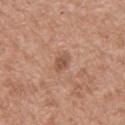biopsy_status: not biopsied; imaged during a skin examination
lesion_size:
  long_diameter_mm_approx: 2.5
patient:
  sex: male
  age_approx: 80
site: back
image:
  source: total-body photography crop
  field_of_view_mm: 15